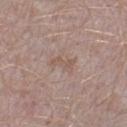Assessment:
This lesion was catalogued during total-body skin photography and was not selected for biopsy.
Background:
A male subject roughly 40 years of age. The lesion is located on the right lower leg. Longest diameter approximately 3 mm. A lesion tile, about 15 mm wide, cut from a 3D total-body photograph. Captured under white-light illumination.